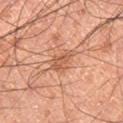Q: Was a biopsy performed?
A: catalogued during a skin exam; not biopsied
Q: What is the imaging modality?
A: 15 mm crop, total-body photography
Q: What is the anatomic site?
A: the right thigh
Q: What are the patient's age and sex?
A: male, aged 58 to 62
Q: Illumination type?
A: cross-polarized